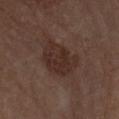illumination = cross-polarized; anatomic site = the arm; subject = female, approximately 70 years of age; image = total-body-photography crop, ~15 mm field of view; diameter = about 5 mm.